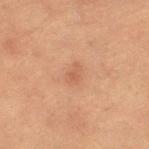Recorded during total-body skin imaging; not selected for excision or biopsy.
Located on the right thigh.
A female subject, in their 80s.
A close-up tile cropped from a whole-body skin photograph, about 15 mm across.